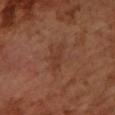workup: total-body-photography surveillance lesion; no biopsy
image: 15 mm crop, total-body photography
patient: female, aged 68 to 72
anatomic site: the left forearm
tile lighting: cross-polarized illumination